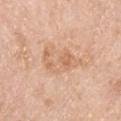Captured during whole-body skin photography for melanoma surveillance; the lesion was not biopsied.
Measured at roughly 5.5 mm in maximum diameter.
The patient is a female aged 68 to 72.
Automated tile analysis of the lesion measured an outline eccentricity of about 0.85 (0 = round, 1 = elongated) and two-axis asymmetry of about 0.45.
The lesion is located on the right lower leg.
A lesion tile, about 15 mm wide, cut from a 3D total-body photograph.